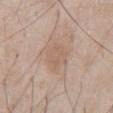{"biopsy_status": "not biopsied; imaged during a skin examination", "automated_metrics": {"cielab_L": 60, "cielab_a": 16, "cielab_b": 28, "vs_skin_darker_L": 6.0, "nevus_likeness_0_100": 0, "lesion_detection_confidence_0_100": 100}, "site": "abdomen", "patient": {"sex": "male", "age_approx": 60}, "lighting": "white-light", "image": {"source": "total-body photography crop", "field_of_view_mm": 15}, "lesion_size": {"long_diameter_mm_approx": 3.5}}